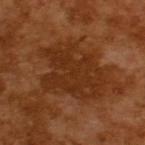<record>
<biopsy_status>not biopsied; imaged during a skin examination</biopsy_status>
<image>
  <source>total-body photography crop</source>
  <field_of_view_mm>15</field_of_view_mm>
</image>
<lighting>cross-polarized</lighting>
<patient>
  <sex>male</sex>
  <age_approx>65</age_approx>
</patient>
<lesion_size>
  <long_diameter_mm_approx>7.0</long_diameter_mm_approx>
</lesion_size>
<automated_metrics>
  <cielab_L>30</cielab_L>
  <cielab_a>23</cielab_a>
  <cielab_b>33</cielab_b>
  <vs_skin_darker_L>7.0</vs_skin_darker_L>
  <vs_skin_contrast_norm>6.5</vs_skin_contrast_norm>
  <color_variation_0_10>2.5</color_variation_0_10>
  <peripheral_color_asymmetry>1.0</peripheral_color_asymmetry>
</automated_metrics>
</record>